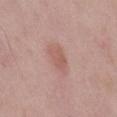Q: Was a biopsy performed?
A: total-body-photography surveillance lesion; no biopsy